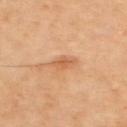Recorded during total-body skin imaging; not selected for excision or biopsy.